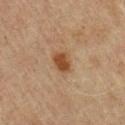The lesion was photographed on a routine skin check and not biopsied; there is no pathology result.
This image is a 15 mm lesion crop taken from a total-body photograph.
The total-body-photography lesion software estimated a footprint of about 4.5 mm², an outline eccentricity of about 0.65 (0 = round, 1 = elongated), and two-axis asymmetry of about 0.2.
The patient is a male in their 60s.
This is a cross-polarized tile.
Measured at roughly 2.5 mm in maximum diameter.